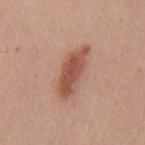Imaged during a routine full-body skin examination; the lesion was not biopsied and no histopathology is available. Measured at roughly 6 mm in maximum diameter. Imaged with white-light lighting. A male patient aged 28 to 32. A 15 mm close-up tile from a total-body photography series done for melanoma screening. Automated image analysis of the tile measured a footprint of about 11 mm² and an outline eccentricity of about 0.9 (0 = round, 1 = elongated). The analysis additionally found a border-irregularity rating of about 3.5/10, internal color variation of about 4.5 on a 0–10 scale, and radial color variation of about 1.5. Located on the back.